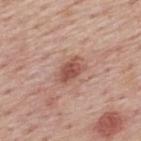Q: Is there a histopathology result?
A: imaged on a skin check; not biopsied
Q: What kind of image is this?
A: ~15 mm crop, total-body skin-cancer survey
Q: Where on the body is the lesion?
A: the upper back
Q: Illumination type?
A: white-light illumination
Q: Who is the patient?
A: male, about 75 years old